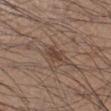Impression:
Captured during whole-body skin photography for melanoma surveillance; the lesion was not biopsied.
Acquisition and patient details:
On the left lower leg. An algorithmic analysis of the crop reported a lesion area of about 4 mm² and a shape eccentricity near 0.75. Captured under white-light illumination. Longest diameter approximately 2.5 mm. A 15 mm close-up tile from a total-body photography series done for melanoma screening. The patient is a male in their mid-30s.